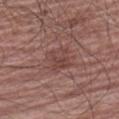Recorded during total-body skin imaging; not selected for excision or biopsy.
A region of skin cropped from a whole-body photographic capture, roughly 15 mm wide.
On the left upper arm.
About 3.5 mm across.
A male subject, in their mid-60s.